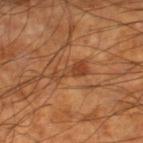subject=male, aged around 60 | TBP lesion metrics=a lesion area of about 6 mm², a shape eccentricity near 0.95, and two-axis asymmetry of about 0.35; a mean CIELAB color near L≈43 a*≈24 b*≈35 and a normalized lesion–skin contrast near 6.5; a border-irregularity index near 4.5/10, a color-variation rating of about 2.5/10, and peripheral color asymmetry of about 0.5 | lighting=cross-polarized | imaging modality=total-body-photography crop, ~15 mm field of view | anatomic site=the right lower leg | diameter=about 4.5 mm.